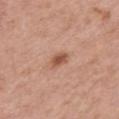* location — the upper back
* diameter — about 2.5 mm
* automated metrics — a lesion area of about 3.5 mm², an outline eccentricity of about 0.8 (0 = round, 1 = elongated), and two-axis asymmetry of about 0.25
* subject — female, in their mid- to late 60s
* tile lighting — white-light illumination
* image source — 15 mm crop, total-body photography